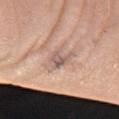Assessment:
Part of a total-body skin-imaging series; this lesion was reviewed on a skin check and was not flagged for biopsy.
Clinical summary:
A female subject, in their mid-70s. A lesion tile, about 15 mm wide, cut from a 3D total-body photograph. From the right forearm.